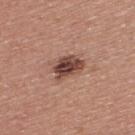Assessment: The lesion was tiled from a total-body skin photograph and was not biopsied. Image and clinical context: The patient is a male aged 38 to 42. This is a white-light tile. On the upper back. A region of skin cropped from a whole-body photographic capture, roughly 15 mm wide. Approximately 3.5 mm at its widest. Automated tile analysis of the lesion measured an average lesion color of about L≈45 a*≈21 b*≈25 (CIELAB) and roughly 15 lightness units darker than nearby skin. The software also gave a classifier nevus-likeness of about 85/100 and a detector confidence of about 100 out of 100 that the crop contains a lesion.